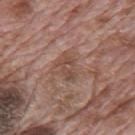Assessment: Part of a total-body skin-imaging series; this lesion was reviewed on a skin check and was not flagged for biopsy. Clinical summary: The lesion is located on the mid back. The subject is a male aged 68 to 72. A 15 mm close-up extracted from a 3D total-body photography capture.